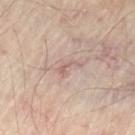<tbp_lesion>
  <image>
    <source>total-body photography crop</source>
    <field_of_view_mm>15</field_of_view_mm>
  </image>
  <patient>
    <age_approx>55</age_approx>
  </patient>
  <site>leg</site>
  <lesion_size>
    <long_diameter_mm_approx>3.0</long_diameter_mm_approx>
  </lesion_size>
  <lighting>cross-polarized</lighting>
</tbp_lesion>